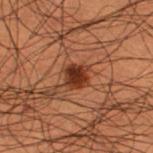Q: Was a biopsy performed?
A: no biopsy performed (imaged during a skin exam)
Q: What kind of image is this?
A: ~15 mm crop, total-body skin-cancer survey
Q: What is the anatomic site?
A: the leg
Q: Who is the patient?
A: male, about 50 years old
Q: How was the tile lit?
A: cross-polarized illumination
Q: What is the lesion's diameter?
A: ~2.5 mm (longest diameter)